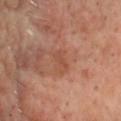image source: 15 mm crop, total-body photography
body site: the chest
lesion diameter: ~3 mm (longest diameter)
subject: male, aged approximately 65
automated lesion analysis: an area of roughly 4 mm², an outline eccentricity of about 0.85 (0 = round, 1 = elongated), and a shape-asymmetry score of about 0.25 (0 = symmetric); a nevus-likeness score of about 0/100 and a detector confidence of about 100 out of 100 that the crop contains a lesion
lighting: cross-polarized illumination